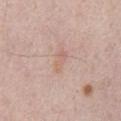The lesion was photographed on a routine skin check and not biopsied; there is no pathology result. Imaged with white-light lighting. A male patient roughly 65 years of age. About 3 mm across. A roughly 15 mm field-of-view crop from a total-body skin photograph. Located on the abdomen. An algorithmic analysis of the crop reported a footprint of about 3 mm² and a shape-asymmetry score of about 0.4 (0 = symmetric). And it measured a mean CIELAB color near L≈63 a*≈19 b*≈26, roughly 6 lightness units darker than nearby skin, and a normalized border contrast of about 4.5. The software also gave an automated nevus-likeness rating near 0 out of 100.